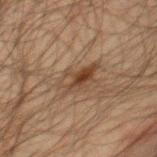No biopsy was performed on this lesion — it was imaged during a full skin examination and was not determined to be concerning.
Located on the front of the torso.
The tile uses cross-polarized illumination.
The lesion-visualizer software estimated an area of roughly 8.5 mm², an outline eccentricity of about 0.85 (0 = round, 1 = elongated), and two-axis asymmetry of about 0.35. The software also gave a normalized border contrast of about 7.5. And it measured a border-irregularity index near 5/10 and peripheral color asymmetry of about 2.5. It also reported a classifier nevus-likeness of about 70/100 and a detector confidence of about 100 out of 100 that the crop contains a lesion.
A lesion tile, about 15 mm wide, cut from a 3D total-body photograph.
Measured at roughly 4.5 mm in maximum diameter.
A male subject, aged around 60.